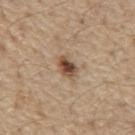notes: catalogued during a skin exam; not biopsied
acquisition: total-body-photography crop, ~15 mm field of view
lighting: white-light
lesion size: ≈3 mm
site: the front of the torso
patient: male, approximately 70 years of age